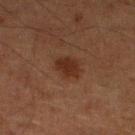No biopsy was performed on this lesion — it was imaged during a full skin examination and was not determined to be concerning.
Cropped from a whole-body photographic skin survey; the tile spans about 15 mm.
Located on the left lower leg.
A male patient aged around 75.